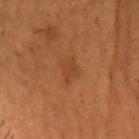notes: total-body-photography surveillance lesion; no biopsy | image-analysis metrics: a lesion color around L≈33 a*≈20 b*≈30 in CIELAB and a lesion-to-skin contrast of about 4.5 (normalized; higher = more distinct); a within-lesion color-variation index near 1.5/10 and a peripheral color-asymmetry measure near 0.5; a nevus-likeness score of about 0/100 and a detector confidence of about 100 out of 100 that the crop contains a lesion | subject: male, in their 50s | image: 15 mm crop, total-body photography | body site: the head or neck | lighting: cross-polarized illumination.